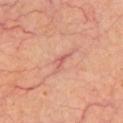| feature | finding |
|---|---|
| biopsy status | no biopsy performed (imaged during a skin exam) |
| acquisition | ~15 mm tile from a whole-body skin photo |
| site | the front of the torso |
| subject | male, in their 60s |